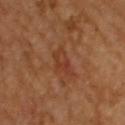lesion_size:
  long_diameter_mm_approx: 3.5
automated_metrics:
  cielab_L: 40
  cielab_a: 26
  cielab_b: 33
  vs_skin_darker_L: 6.0
  vs_skin_contrast_norm: 5.5
  border_irregularity_0_10: 4.0
  color_variation_0_10: 4.0
  peripheral_color_asymmetry: 1.5
lighting: cross-polarized
image:
  source: total-body photography crop
  field_of_view_mm: 15
site: upper back